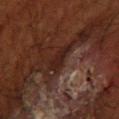| feature | finding |
|---|---|
| biopsy status | imaged on a skin check; not biopsied |
| patient | female, approximately 80 years of age |
| anatomic site | the left forearm |
| acquisition | 15 mm crop, total-body photography |
| diameter | ~3 mm (longest diameter) |
| TBP lesion metrics | an average lesion color of about L≈15 a*≈15 b*≈16 (CIELAB), about 6 CIELAB-L* units darker than the surrounding skin, and a normalized lesion–skin contrast near 9; a classifier nevus-likeness of about 0/100 and lesion-presence confidence of about 5/100 |
| lighting | cross-polarized |